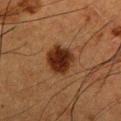Q: How was this image acquired?
A: total-body-photography crop, ~15 mm field of view
Q: What is the anatomic site?
A: the left upper arm
Q: What lighting was used for the tile?
A: cross-polarized illumination
Q: Patient demographics?
A: male, aged 48 to 52